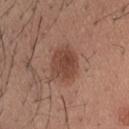No biopsy was performed on this lesion — it was imaged during a full skin examination and was not determined to be concerning. Captured under white-light illumination. Cropped from a total-body skin-imaging series; the visible field is about 15 mm. An algorithmic analysis of the crop reported an area of roughly 11 mm², a shape eccentricity near 0.55, and a shape-asymmetry score of about 0.15 (0 = symmetric). The software also gave an average lesion color of about L≈44 a*≈22 b*≈27 (CIELAB), about 10 CIELAB-L* units darker than the surrounding skin, and a normalized border contrast of about 8. Located on the head or neck. A male subject, approximately 30 years of age.